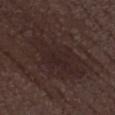Assessment:
The lesion was photographed on a routine skin check and not biopsied; there is no pathology result.
Image and clinical context:
The subject is a male aged around 70. A 15 mm crop from a total-body photograph taken for skin-cancer surveillance. On the right lower leg.